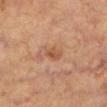{"biopsy_status": "not biopsied; imaged during a skin examination", "lighting": "cross-polarized", "site": "left lower leg", "image": {"source": "total-body photography crop", "field_of_view_mm": 15}, "patient": {"sex": "female", "age_approx": 60}, "automated_metrics": {"area_mm2_approx": 3.0, "vs_skin_darker_L": 8.0, "nevus_likeness_0_100": 0, "lesion_detection_confidence_0_100": 100}, "lesion_size": {"long_diameter_mm_approx": 2.5}}